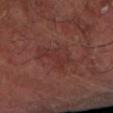A male patient, roughly 65 years of age. The lesion is located on the left forearm. A close-up tile cropped from a whole-body skin photograph, about 15 mm across.